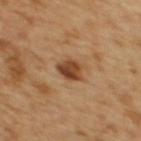Imaged during a routine full-body skin examination; the lesion was not biopsied and no histopathology is available. The tile uses cross-polarized illumination. Automated image analysis of the tile measured a lesion area of about 5.5 mm² and two-axis asymmetry of about 0.25. The analysis additionally found a lesion color around L≈45 a*≈22 b*≈35 in CIELAB, about 14 CIELAB-L* units darker than the surrounding skin, and a lesion-to-skin contrast of about 10.5 (normalized; higher = more distinct). The analysis additionally found a lesion-detection confidence of about 100/100. A region of skin cropped from a whole-body photographic capture, roughly 15 mm wide. A female patient, aged approximately 55. The lesion's longest dimension is about 3 mm. Located on the upper back.